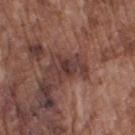<tbp_lesion>
  <biopsy_status>not biopsied; imaged during a skin examination</biopsy_status>
</tbp_lesion>